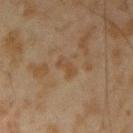{"biopsy_status": "not biopsied; imaged during a skin examination"}